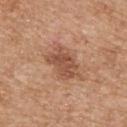The lesion's longest dimension is about 4.5 mm. A female subject about 70 years old. Imaged with white-light lighting. From the upper back. Cropped from a total-body skin-imaging series; the visible field is about 15 mm.